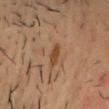Assessment: The lesion was tiled from a total-body skin photograph and was not biopsied. Background: This is a cross-polarized tile. The lesion is on the chest. A close-up tile cropped from a whole-body skin photograph, about 15 mm across. The lesion-visualizer software estimated an eccentricity of roughly 0.9 and a shape-asymmetry score of about 0.25 (0 = symmetric). It also reported an average lesion color of about L≈42 a*≈19 b*≈32 (CIELAB), a lesion–skin lightness drop of about 8, and a lesion-to-skin contrast of about 8 (normalized; higher = more distinct). A male subject aged 33–37. Approximately 3 mm at its widest.